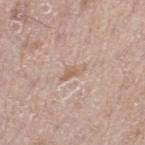Q: Was a biopsy performed?
A: no biopsy performed (imaged during a skin exam)
Q: Who is the patient?
A: male, in their mid-60s
Q: What did automated image analysis measure?
A: a footprint of about 3 mm²; an average lesion color of about L≈61 a*≈17 b*≈27 (CIELAB), roughly 8 lightness units darker than nearby skin, and a lesion-to-skin contrast of about 5.5 (normalized; higher = more distinct); a nevus-likeness score of about 0/100 and lesion-presence confidence of about 90/100
Q: Lesion size?
A: ~3 mm (longest diameter)
Q: What kind of image is this?
A: total-body-photography crop, ~15 mm field of view
Q: Lesion location?
A: the left thigh
Q: How was the tile lit?
A: white-light illumination